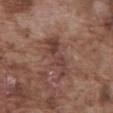Part of a total-body skin-imaging series; this lesion was reviewed on a skin check and was not flagged for biopsy.
This is a white-light tile.
Located on the abdomen.
About 5 mm across.
A 15 mm crop from a total-body photograph taken for skin-cancer surveillance.
The patient is a male aged 73 to 77.